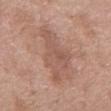Q: Is there a histopathology result?
A: imaged on a skin check; not biopsied
Q: What is the anatomic site?
A: the chest
Q: What did automated image analysis measure?
A: a lesion area of about 19 mm², an eccentricity of roughly 0.9, and a shape-asymmetry score of about 0.45 (0 = symmetric); border irregularity of about 6 on a 0–10 scale and a color-variation rating of about 2.5/10
Q: How was the tile lit?
A: white-light illumination
Q: What is the lesion's diameter?
A: ~8.5 mm (longest diameter)
Q: What is the imaging modality?
A: ~15 mm tile from a whole-body skin photo
Q: Who is the patient?
A: female, approximately 75 years of age